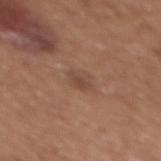* follow-up — total-body-photography surveillance lesion; no biopsy
* size — ≈2.5 mm
* location — the chest
* image — ~15 mm crop, total-body skin-cancer survey
* subject — female, approximately 35 years of age
* illumination — white-light illumination